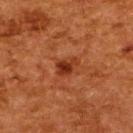The lesion is located on the upper back. Imaged with cross-polarized lighting. The patient is a female approximately 50 years of age. Cropped from a total-body skin-imaging series; the visible field is about 15 mm.